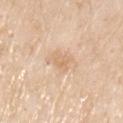{
  "biopsy_status": "not biopsied; imaged during a skin examination",
  "patient": {
    "sex": "male",
    "age_approx": 80
  },
  "image": {
    "source": "total-body photography crop",
    "field_of_view_mm": 15
  },
  "site": "chest"
}